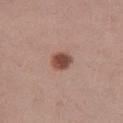  biopsy_status: not biopsied; imaged during a skin examination
  patient:
    sex: male
    age_approx: 50
  automated_metrics:
    area_mm2_approx: 5.0
    shape_asymmetry: 0.15
  image:
    source: total-body photography crop
    field_of_view_mm: 15
  site: left lower leg